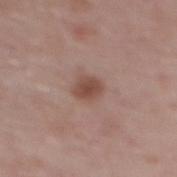follow-up: total-body-photography surveillance lesion; no biopsy
imaging modality: ~15 mm crop, total-body skin-cancer survey
body site: the upper back
diameter: ≈2.5 mm
subject: female, roughly 65 years of age
automated lesion analysis: a nevus-likeness score of about 90/100 and lesion-presence confidence of about 100/100